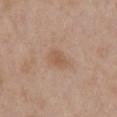Captured during whole-body skin photography for melanoma surveillance; the lesion was not biopsied. An algorithmic analysis of the crop reported an average lesion color of about L≈55 a*≈18 b*≈30 (CIELAB), about 7 CIELAB-L* units darker than the surrounding skin, and a normalized lesion–skin contrast near 5.5. The software also gave a border-irregularity index near 2.5/10 and internal color variation of about 2 on a 0–10 scale. This image is a 15 mm lesion crop taken from a total-body photograph. A female subject, aged 38–42. On the chest. The lesion's longest dimension is about 2.5 mm. Imaged with white-light lighting.